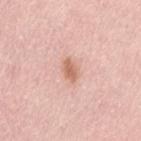Impression: Captured during whole-body skin photography for melanoma surveillance; the lesion was not biopsied.